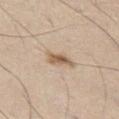Q: What is the imaging modality?
A: total-body-photography crop, ~15 mm field of view
Q: What are the patient's age and sex?
A: female, roughly 60 years of age
Q: Lesion location?
A: the leg
Q: How large is the lesion?
A: ~3.5 mm (longest diameter)